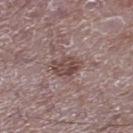Clinical impression:
Imaged during a routine full-body skin examination; the lesion was not biopsied and no histopathology is available.
Background:
A male subject, aged 48 to 52. From the right lower leg. Imaged with white-light lighting. A region of skin cropped from a whole-body photographic capture, roughly 15 mm wide.